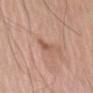Findings:
– tile lighting: white-light
– image-analysis metrics: an average lesion color of about L≈56 a*≈21 b*≈29 (CIELAB), a lesion–skin lightness drop of about 8, and a lesion-to-skin contrast of about 5.5 (normalized; higher = more distinct); a border-irregularity index near 4.5/10, internal color variation of about 2.5 on a 0–10 scale, and radial color variation of about 1; a classifier nevus-likeness of about 0/100
– site: the left upper arm
– image source: total-body-photography crop, ~15 mm field of view
– lesion size: ~3 mm (longest diameter)
– patient: male, aged 58 to 62